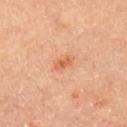The lesion was photographed on a routine skin check and not biopsied; there is no pathology result.
The patient is a male aged 63–67.
Longest diameter approximately 2.5 mm.
This is a cross-polarized tile.
The lesion is located on the right upper arm.
A 15 mm crop from a total-body photograph taken for skin-cancer surveillance.